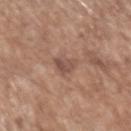Imaged during a routine full-body skin examination; the lesion was not biopsied and no histopathology is available.
A 15 mm close-up extracted from a 3D total-body photography capture.
The total-body-photography lesion software estimated a footprint of about 4.5 mm², a shape eccentricity near 0.65, and a symmetry-axis asymmetry near 0.3. The analysis additionally found a classifier nevus-likeness of about 0/100 and a lesion-detection confidence of about 100/100.
A male patient, in their mid-70s.
The lesion is on the left upper arm.
Captured under white-light illumination.